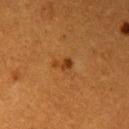• notes — catalogued during a skin exam; not biopsied
• patient — female, approximately 55 years of age
• imaging modality — ~15 mm tile from a whole-body skin photo
• size — about 2.5 mm
• site — the arm